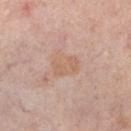Q: Is there a histopathology result?
A: catalogued during a skin exam; not biopsied
Q: How was this image acquired?
A: ~15 mm crop, total-body skin-cancer survey
Q: Lesion size?
A: ≈3.5 mm
Q: Automated lesion metrics?
A: about 5 CIELAB-L* units darker than the surrounding skin and a normalized border contrast of about 5; a nevus-likeness score of about 0/100 and a lesion-detection confidence of about 100/100
Q: Who is the patient?
A: female, aged approximately 50
Q: Lesion location?
A: the leg
Q: Illumination type?
A: cross-polarized